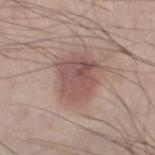<lesion>
  <biopsy_status>not biopsied; imaged during a skin examination</biopsy_status>
  <patient>
    <sex>male</sex>
    <age_approx>60</age_approx>
  </patient>
  <image>
    <source>total-body photography crop</source>
    <field_of_view_mm>15</field_of_view_mm>
  </image>
  <automated_metrics>
    <eccentricity>0.55</eccentricity>
    <shape_asymmetry>0.2</shape_asymmetry>
  </automated_metrics>
  <lighting>white-light</lighting>
  <lesion_size>
    <long_diameter_mm_approx>5.0</long_diameter_mm_approx>
  </lesion_size>
  <site>left thigh</site>
</lesion>